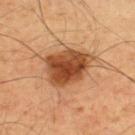{
  "image": {
    "source": "total-body photography crop",
    "field_of_view_mm": 15
  },
  "site": "upper back",
  "lesion_size": {
    "long_diameter_mm_approx": 5.5
  },
  "lighting": "cross-polarized",
  "automated_metrics": {
    "border_irregularity_0_10": 2.0,
    "color_variation_0_10": 5.5,
    "peripheral_color_asymmetry": 2.0,
    "nevus_likeness_0_100": 95,
    "lesion_detection_confidence_0_100": 100
  },
  "patient": {
    "sex": "male",
    "age_approx": 50
  }
}